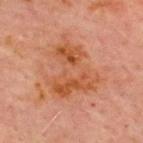This lesion was catalogued during total-body skin photography and was not selected for biopsy.
The lesion is on the chest.
A male patient, aged 68 to 72.
Cropped from a whole-body photographic skin survey; the tile spans about 15 mm.